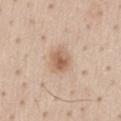{
  "biopsy_status": "not biopsied; imaged during a skin examination",
  "image": {
    "source": "total-body photography crop",
    "field_of_view_mm": 15
  },
  "site": "front of the torso",
  "patient": {
    "sex": "male",
    "age_approx": 45
  }
}